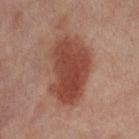Clinical impression:
This lesion was catalogued during total-body skin photography and was not selected for biopsy.
Acquisition and patient details:
Located on the right thigh. The lesion's longest dimension is about 8 mm. A lesion tile, about 15 mm wide, cut from a 3D total-body photograph. This is a cross-polarized tile. The subject is a female roughly 55 years of age.